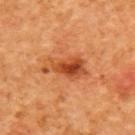Part of a total-body skin-imaging series; this lesion was reviewed on a skin check and was not flagged for biopsy. The lesion-visualizer software estimated a mean CIELAB color near L≈51 a*≈32 b*≈43, roughly 13 lightness units darker than nearby skin, and a normalized lesion–skin contrast near 8.5. The analysis additionally found a classifier nevus-likeness of about 95/100 and lesion-presence confidence of about 100/100. Captured under cross-polarized illumination. The lesion is located on the upper back. A female subject, aged around 40. Measured at roughly 5.5 mm in maximum diameter. A lesion tile, about 15 mm wide, cut from a 3D total-body photograph.